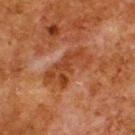Captured during whole-body skin photography for melanoma surveillance; the lesion was not biopsied. On the upper back. A 15 mm close-up extracted from a 3D total-body photography capture. The subject is a male approximately 80 years of age. The tile uses cross-polarized illumination. Approximately 6.5 mm at its widest. The lesion-visualizer software estimated an average lesion color of about L≈34 a*≈23 b*≈30 (CIELAB), roughly 7 lightness units darker than nearby skin, and a normalized border contrast of about 7.